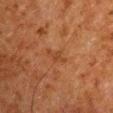A male subject, aged 58 to 62.
Automated image analysis of the tile measured a lesion area of about 3 mm² and a shape-asymmetry score of about 0.45 (0 = symmetric). It also reported border irregularity of about 5.5 on a 0–10 scale, a within-lesion color-variation index near 0/10, and peripheral color asymmetry of about 0. The analysis additionally found a classifier nevus-likeness of about 0/100.
A region of skin cropped from a whole-body photographic capture, roughly 15 mm wide.
Located on the arm.
The tile uses cross-polarized illumination.